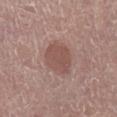Context: The lesion is located on the right lower leg. A male patient aged 78 to 82. Imaged with white-light lighting. Measured at roughly 4 mm in maximum diameter. The lesion-visualizer software estimated a lesion color around L≈51 a*≈20 b*≈23 in CIELAB and a lesion–skin lightness drop of about 9. The software also gave a classifier nevus-likeness of about 55/100 and lesion-presence confidence of about 100/100. A roughly 15 mm field-of-view crop from a total-body skin photograph.